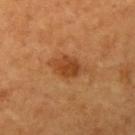follow-up: no biopsy performed (imaged during a skin exam)
patient: female, aged 58 to 62
tile lighting: cross-polarized illumination
lesion size: ≈4.5 mm
anatomic site: the left upper arm
image source: ~15 mm crop, total-body skin-cancer survey